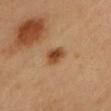The lesion was photographed on a routine skin check and not biopsied; there is no pathology result. The patient is a female about 35 years old. A 15 mm close-up extracted from a 3D total-body photography capture. Located on the head or neck. The lesion's longest dimension is about 3 mm.